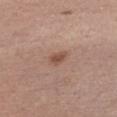- biopsy status — catalogued during a skin exam; not biopsied
- diameter — ≈2.5 mm
- tile lighting — white-light illumination
- acquisition — 15 mm crop, total-body photography
- patient — male, aged approximately 30
- TBP lesion metrics — a border-irregularity index near 2.5/10 and peripheral color asymmetry of about 0.5